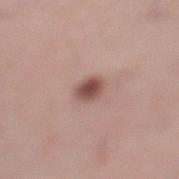Assessment: The lesion was tiled from a total-body skin photograph and was not biopsied. Acquisition and patient details: A roughly 15 mm field-of-view crop from a total-body skin photograph. A female subject, about 40 years old. The lesion is located on the left thigh. Automated image analysis of the tile measured an eccentricity of roughly 0.55 and a shape-asymmetry score of about 0.15 (0 = symmetric). It also reported roughly 14 lightness units darker than nearby skin and a normalized lesion–skin contrast near 10. Approximately 2.5 mm at its widest. The tile uses white-light illumination.